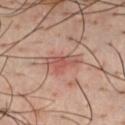{
  "biopsy_status": "not biopsied; imaged during a skin examination",
  "patient": {
    "sex": "male",
    "age_approx": 40
  },
  "lighting": "cross-polarized",
  "lesion_size": {
    "long_diameter_mm_approx": 4.0
  },
  "image": {
    "source": "total-body photography crop",
    "field_of_view_mm": 15
  },
  "site": "chest"
}